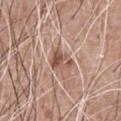  biopsy_status: not biopsied; imaged during a skin examination
  site: chest
  lighting: white-light
  automated_metrics:
    area_mm2_approx: 5.5
    eccentricity: 0.6
    shape_asymmetry: 0.35
    border_irregularity_0_10: 3.5
    color_variation_0_10: 7.0
    peripheral_color_asymmetry: 3.0
  lesion_size:
    long_diameter_mm_approx: 3.0
  patient:
    sex: male
    age_approx: 60
  image:
    source: total-body photography crop
    field_of_view_mm: 15